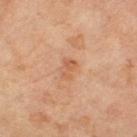{"site": "left thigh", "automated_metrics": {"cielab_L": 57, "cielab_a": 23, "cielab_b": 36, "border_irregularity_0_10": 4.5, "color_variation_0_10": 1.5, "peripheral_color_asymmetry": 0.5}, "lesion_size": {"long_diameter_mm_approx": 2.5}, "lighting": "cross-polarized", "patient": {"sex": "female", "age_approx": 65}, "image": {"source": "total-body photography crop", "field_of_view_mm": 15}}